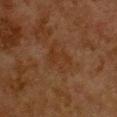Case summary:
– workup · total-body-photography surveillance lesion; no biopsy